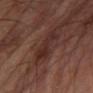Assessment: Captured during whole-body skin photography for melanoma surveillance; the lesion was not biopsied. Image and clinical context: On the left forearm. A male subject about 65 years old. Automated tile analysis of the lesion measured a footprint of about 9 mm², an outline eccentricity of about 0.75 (0 = round, 1 = elongated), and a shape-asymmetry score of about 0.3 (0 = symmetric). The software also gave an average lesion color of about L≈27 a*≈19 b*≈20 (CIELAB) and a lesion-to-skin contrast of about 6.5 (normalized; higher = more distinct). And it measured border irregularity of about 4 on a 0–10 scale, a within-lesion color-variation index near 3/10, and radial color variation of about 1. Approximately 4 mm at its widest. A 15 mm close-up extracted from a 3D total-body photography capture.